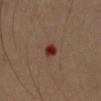workup = no biopsy performed (imaged during a skin exam); imaging modality = total-body-photography crop, ~15 mm field of view; patient = male, aged 48–52; illumination = cross-polarized; anatomic site = the left arm.